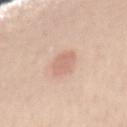Impression:
Recorded during total-body skin imaging; not selected for excision or biopsy.
Background:
Longest diameter approximately 3.5 mm. Imaged with white-light lighting. The subject is a female aged 48–52. On the mid back. An algorithmic analysis of the crop reported a lesion area of about 6 mm² and two-axis asymmetry of about 0.25. It also reported an average lesion color of about L≈67 a*≈20 b*≈28 (CIELAB) and a lesion-to-skin contrast of about 5.5 (normalized; higher = more distinct). The analysis additionally found a border-irregularity rating of about 2.5/10 and a within-lesion color-variation index near 1/10. It also reported a nevus-likeness score of about 25/100. Cropped from a total-body skin-imaging series; the visible field is about 15 mm.